On the mid back; the subject is a male about 35 years old; a roughly 15 mm field-of-view crop from a total-body skin photograph: 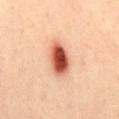Histopathologically confirmed as a junctional melanocytic nevus.